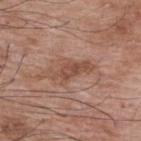• notes · imaged on a skin check; not biopsied
• body site · the upper back
• acquisition · 15 mm crop, total-body photography
• subject · male, approximately 70 years of age
• automated metrics · a footprint of about 9 mm² and a symmetry-axis asymmetry near 0.3; a mean CIELAB color near L≈50 a*≈21 b*≈28, roughly 8 lightness units darker than nearby skin, and a normalized lesion–skin contrast near 6.5; border irregularity of about 5 on a 0–10 scale, a color-variation rating of about 2.5/10, and a peripheral color-asymmetry measure near 0.5; a nevus-likeness score of about 0/100 and a lesion-detection confidence of about 95/100